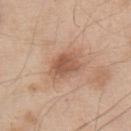Notes:
– workup: total-body-photography surveillance lesion; no biopsy
– body site: the upper back
– patient: male, about 55 years old
– image: total-body-photography crop, ~15 mm field of view
– image-analysis metrics: a mean CIELAB color near L≈56 a*≈21 b*≈31, roughly 11 lightness units darker than nearby skin, and a normalized border contrast of about 7.5; an automated nevus-likeness rating near 70 out of 100 and lesion-presence confidence of about 100/100
– lesion size: ~4.5 mm (longest diameter)